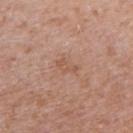biopsy_status: not biopsied; imaged during a skin examination
lesion_size:
  long_diameter_mm_approx: 2.5
automated_metrics:
  vs_skin_darker_L: 6.0
  vs_skin_contrast_norm: 5.0
site: right upper arm
image:
  source: total-body photography crop
  field_of_view_mm: 15
lighting: white-light
patient:
  sex: female
  age_approx: 40